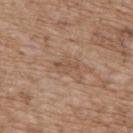Notes:
– workup — total-body-photography surveillance lesion; no biopsy
– site — the upper back
– lesion diameter — ≈3 mm
– patient — male, aged 68 to 72
– tile lighting — white-light
– acquisition — total-body-photography crop, ~15 mm field of view